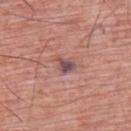Recorded during total-body skin imaging; not selected for excision or biopsy. A 15 mm close-up extracted from a 3D total-body photography capture. This is a white-light tile. The patient is a male aged 58 to 62. The lesion is on the upper back. The lesion-visualizer software estimated a border-irregularity index near 3/10 and peripheral color asymmetry of about 1. Measured at roughly 2.5 mm in maximum diameter.